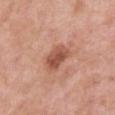Clinical impression: No biopsy was performed on this lesion — it was imaged during a full skin examination and was not determined to be concerning. Background: The lesion's longest dimension is about 3.5 mm. A 15 mm close-up extracted from a 3D total-body photography capture. A male patient, aged approximately 55. Located on the right upper arm.